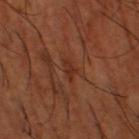Clinical impression:
No biopsy was performed on this lesion — it was imaged during a full skin examination and was not determined to be concerning.
Background:
A close-up tile cropped from a whole-body skin photograph, about 15 mm across. A male subject about 65 years old. This is a cross-polarized tile. From the leg. Measured at roughly 2.5 mm in maximum diameter.